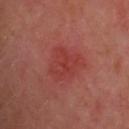workup: imaged on a skin check; not biopsied | image: ~15 mm crop, total-body skin-cancer survey | site: the upper back | lighting: cross-polarized | patient: female, aged 53–57 | automated metrics: a border-irregularity index near 2.5/10 and a within-lesion color-variation index near 3/10; a nevus-likeness score of about 15/100 and a detector confidence of about 100 out of 100 that the crop contains a lesion.